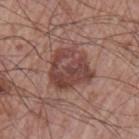The lesion was tiled from a total-body skin photograph and was not biopsied.
The lesion is located on the left upper arm.
The total-body-photography lesion software estimated a footprint of about 19 mm², an outline eccentricity of about 0.1 (0 = round, 1 = elongated), and a shape-asymmetry score of about 0.25 (0 = symmetric).
About 5 mm across.
A 15 mm close-up tile from a total-body photography series done for melanoma screening.
A male subject, in their mid-60s.
Captured under white-light illumination.